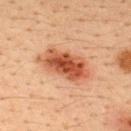An algorithmic analysis of the crop reported an area of roughly 16 mm², an eccentricity of roughly 0.85, and a shape-asymmetry score of about 0.2 (0 = symmetric). The analysis additionally found a mean CIELAB color near L≈50 a*≈27 b*≈35, roughly 15 lightness units darker than nearby skin, and a normalized border contrast of about 10.
On the upper back.
Cropped from a total-body skin-imaging series; the visible field is about 15 mm.
The lesion's longest dimension is about 6.5 mm.
The subject is a male aged approximately 35.